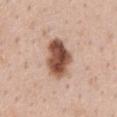Recorded during total-body skin imaging; not selected for excision or biopsy.